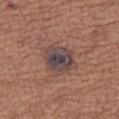No biopsy was performed on this lesion — it was imaged during a full skin examination and was not determined to be concerning. The patient is a female aged 73–77. From the leg. A 15 mm close-up tile from a total-body photography series done for melanoma screening. Longest diameter approximately 4 mm.